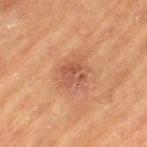Q: Where on the body is the lesion?
A: the left thigh
Q: How large is the lesion?
A: ≈3.5 mm
Q: How was the tile lit?
A: cross-polarized
Q: Who is the patient?
A: male, roughly 85 years of age or older
Q: What is the imaging modality?
A: ~15 mm tile from a whole-body skin photo